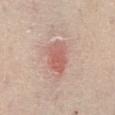workup: no biopsy performed (imaged during a skin exam)
subject: male, aged around 75
diameter: ≈4 mm
imaging modality: ~15 mm tile from a whole-body skin photo
automated lesion analysis: an eccentricity of roughly 0.7 and a shape-asymmetry score of about 0.2 (0 = symmetric); a lesion color around L≈60 a*≈25 b*≈26 in CIELAB and a normalized lesion–skin contrast near 6.5; internal color variation of about 4 on a 0–10 scale
location: the abdomen
tile lighting: white-light illumination